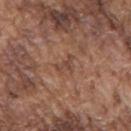<case>
  <biopsy_status>not biopsied; imaged during a skin examination</biopsy_status>
  <lighting>white-light</lighting>
  <site>mid back</site>
  <lesion_size>
    <long_diameter_mm_approx>3.0</long_diameter_mm_approx>
  </lesion_size>
  <patient>
    <sex>male</sex>
    <age_approx>75</age_approx>
  </patient>
  <image>
    <source>total-body photography crop</source>
    <field_of_view_mm>15</field_of_view_mm>
  </image>
  <automated_metrics>
    <area_mm2_approx>3.5</area_mm2_approx>
    <eccentricity>0.85</eccentricity>
    <shape_asymmetry>0.45</shape_asymmetry>
    <cielab_L>45</cielab_L>
    <cielab_a>20</cielab_a>
    <cielab_b>28</cielab_b>
    <vs_skin_darker_L>7.0</vs_skin_darker_L>
    <vs_skin_contrast_norm>5.5</vs_skin_contrast_norm>
    <border_irregularity_0_10>5.0</border_irregularity_0_10>
    <color_variation_0_10>0.5</color_variation_0_10>
    <peripheral_color_asymmetry>0.5</peripheral_color_asymmetry>
    <nevus_likeness_0_100>0</nevus_likeness_0_100>
    <lesion_detection_confidence_0_100>95</lesion_detection_confidence_0_100>
  </automated_metrics>
</case>